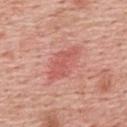Captured during whole-body skin photography for melanoma surveillance; the lesion was not biopsied. This image is a 15 mm lesion crop taken from a total-body photograph. Captured under white-light illumination. Located on the back. The subject is a male aged approximately 60.